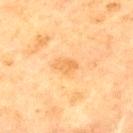This lesion was catalogued during total-body skin photography and was not selected for biopsy.
Cropped from a whole-body photographic skin survey; the tile spans about 15 mm.
The tile uses cross-polarized illumination.
A male patient, approximately 65 years of age.
The recorded lesion diameter is about 3 mm.
An algorithmic analysis of the crop reported a footprint of about 4 mm² and an outline eccentricity of about 0.8 (0 = round, 1 = elongated).
The lesion is located on the chest.